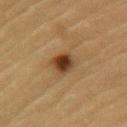Imaged during a routine full-body skin examination; the lesion was not biopsied and no histopathology is available.
The subject is a male in their mid-80s or older.
A roughly 15 mm field-of-view crop from a total-body skin photograph.
The lesion is located on the left upper arm.